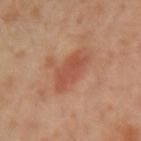workup = no biopsy performed (imaged during a skin exam); size = about 4.5 mm; subject = female, aged 38 to 42; acquisition = 15 mm crop, total-body photography; body site = the left arm; lighting = cross-polarized illumination; image-analysis metrics = an average lesion color of about L≈52 a*≈26 b*≈33 (CIELAB), a lesion–skin lightness drop of about 8, and a normalized border contrast of about 6.